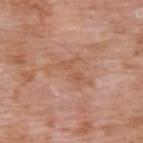* notes: total-body-photography surveillance lesion; no biopsy
* imaging modality: total-body-photography crop, ~15 mm field of view
* size: ≈5.5 mm
* site: the upper back
* automated metrics: a mean CIELAB color near L≈56 a*≈22 b*≈32, about 6 CIELAB-L* units darker than the surrounding skin, and a normalized border contrast of about 5; a border-irregularity rating of about 9/10, internal color variation of about 1 on a 0–10 scale, and a peripheral color-asymmetry measure near 0.5; lesion-presence confidence of about 100/100
* patient: male, in their 60s
* illumination: white-light